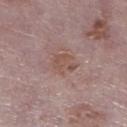Q: Was this lesion biopsied?
A: no biopsy performed (imaged during a skin exam)